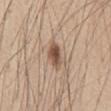lighting — white-light | site — the abdomen | lesion diameter — ~3.5 mm (longest diameter) | patient — male, aged around 45 | image source — 15 mm crop, total-body photography | automated lesion analysis — a footprint of about 5.5 mm², an outline eccentricity of about 0.8 (0 = round, 1 = elongated), and two-axis asymmetry of about 0.25; a mean CIELAB color near L≈53 a*≈17 b*≈28 and a normalized lesion–skin contrast near 9; a color-variation rating of about 5/10 and radial color variation of about 1.5.